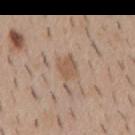Clinical impression: No biopsy was performed on this lesion — it was imaged during a full skin examination and was not determined to be concerning. Acquisition and patient details: A male subject, aged around 40. A lesion tile, about 15 mm wide, cut from a 3D total-body photograph. The lesion is located on the mid back.